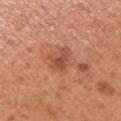notes = total-body-photography surveillance lesion; no biopsy | lighting = white-light | subject = male, in their mid- to late 50s | image = ~15 mm crop, total-body skin-cancer survey | body site = the right upper arm | lesion diameter = ≈3 mm.